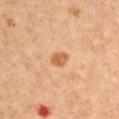{
  "biopsy_status": "not biopsied; imaged during a skin examination",
  "patient": {
    "sex": "female",
    "age_approx": 45
  },
  "automated_metrics": {
    "cielab_L": 61,
    "cielab_a": 24,
    "cielab_b": 41,
    "vs_skin_darker_L": 11.0,
    "border_irregularity_0_10": 1.5,
    "color_variation_0_10": 2.5,
    "peripheral_color_asymmetry": 1.0,
    "nevus_likeness_0_100": 65,
    "lesion_detection_confidence_0_100": 100
  },
  "lesion_size": {
    "long_diameter_mm_approx": 2.0
  },
  "image": {
    "source": "total-body photography crop",
    "field_of_view_mm": 15
  },
  "site": "left upper arm"
}